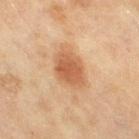Case summary:
* lesion diameter · about 5.5 mm
* patient · female, in their 60s
* lighting · cross-polarized illumination
* body site · the right thigh
* automated lesion analysis · an area of roughly 13 mm², an outline eccentricity of about 0.75 (0 = round, 1 = elongated), and a symmetry-axis asymmetry near 0.2; a border-irregularity index near 2.5/10, a within-lesion color-variation index near 3/10, and a peripheral color-asymmetry measure near 1; an automated nevus-likeness rating near 100 out of 100 and a lesion-detection confidence of about 100/100
* imaging modality · ~15 mm crop, total-body skin-cancer survey